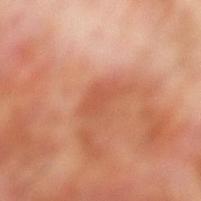Part of a total-body skin-imaging series; this lesion was reviewed on a skin check and was not flagged for biopsy. The recorded lesion diameter is about 4.5 mm. A lesion tile, about 15 mm wide, cut from a 3D total-body photograph. A male patient approximately 70 years of age. The lesion is on the left lower leg. Imaged with cross-polarized lighting. The lesion-visualizer software estimated a border-irregularity index near 4/10. The software also gave a lesion-detection confidence of about 100/100.